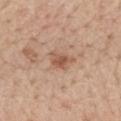Imaged during a routine full-body skin examination; the lesion was not biopsied and no histopathology is available.
Cropped from a whole-body photographic skin survey; the tile spans about 15 mm.
Automated tile analysis of the lesion measured an area of roughly 4.5 mm², a shape eccentricity near 0.8, and a symmetry-axis asymmetry near 0.35. And it measured a lesion color around L≈55 a*≈22 b*≈31 in CIELAB, about 10 CIELAB-L* units darker than the surrounding skin, and a lesion-to-skin contrast of about 7.5 (normalized; higher = more distinct).
The lesion is located on the mid back.
A male subject in their mid- to late 60s.
Captured under white-light illumination.